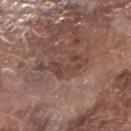Impression: Part of a total-body skin-imaging series; this lesion was reviewed on a skin check and was not flagged for biopsy. Acquisition and patient details: A roughly 15 mm field-of-view crop from a total-body skin photograph. The tile uses white-light illumination. The patient is a male aged around 75. The lesion is on the right forearm. Automated image analysis of the tile measured a lesion area of about 9.5 mm² and an outline eccentricity of about 0.9 (0 = round, 1 = elongated). The software also gave an average lesion color of about L≈43 a*≈19 b*≈23 (CIELAB), a lesion–skin lightness drop of about 8, and a normalized border contrast of about 6.5. It also reported border irregularity of about 8 on a 0–10 scale, a within-lesion color-variation index near 4/10, and peripheral color asymmetry of about 1.5. It also reported an automated nevus-likeness rating near 0 out of 100 and a detector confidence of about 60 out of 100 that the crop contains a lesion. About 6 mm across.